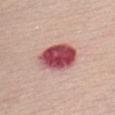Part of a total-body skin-imaging series; this lesion was reviewed on a skin check and was not flagged for biopsy. Located on the chest. The recorded lesion diameter is about 5.5 mm. A female patient, in their mid- to late 50s. A 15 mm close-up tile from a total-body photography series done for melanoma screening. Automated tile analysis of the lesion measured a lesion color around L≈51 a*≈33 b*≈21 in CIELAB. And it measured a border-irregularity rating of about 1.5/10, a within-lesion color-variation index near 9/10, and peripheral color asymmetry of about 3. And it measured an automated nevus-likeness rating near 0 out of 100.